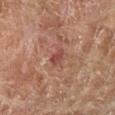Captured during whole-body skin photography for melanoma surveillance; the lesion was not biopsied.